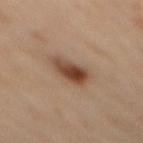Clinical impression:
Captured during whole-body skin photography for melanoma surveillance; the lesion was not biopsied.
Acquisition and patient details:
The lesion is on the mid back. A 15 mm crop from a total-body photograph taken for skin-cancer surveillance. A male subject about 65 years old.